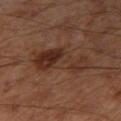Q: Is there a histopathology result?
A: imaged on a skin check; not biopsied
Q: Lesion size?
A: about 7 mm
Q: Patient demographics?
A: male, aged around 55
Q: What is the anatomic site?
A: the right thigh
Q: How was the tile lit?
A: cross-polarized
Q: What did automated image analysis measure?
A: a footprint of about 19 mm² and an outline eccentricity of about 0.9 (0 = round, 1 = elongated); a mean CIELAB color near L≈32 a*≈19 b*≈26 and a lesion-to-skin contrast of about 7 (normalized; higher = more distinct); a border-irregularity rating of about 5/10 and radial color variation of about 2.5
Q: How was this image acquired?
A: ~15 mm tile from a whole-body skin photo